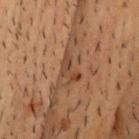follow-up: total-body-photography surveillance lesion; no biopsy
illumination: cross-polarized
site: the head or neck
patient: male, roughly 50 years of age
image: 15 mm crop, total-body photography
size: about 3.5 mm